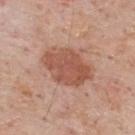- follow-up · no biopsy performed (imaged during a skin exam)
- acquisition · 15 mm crop, total-body photography
- anatomic site · the upper back
- patient · male, aged approximately 60